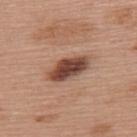Imaged during a routine full-body skin examination; the lesion was not biopsied and no histopathology is available.
On the upper back.
Measured at roughly 5 mm in maximum diameter.
A 15 mm close-up extracted from a 3D total-body photography capture.
A female patient, roughly 60 years of age.
Automated image analysis of the tile measured an area of roughly 9.5 mm², an outline eccentricity of about 0.9 (0 = round, 1 = elongated), and a symmetry-axis asymmetry near 0.2. And it measured a classifier nevus-likeness of about 90/100 and lesion-presence confidence of about 100/100.